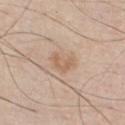Assessment: The lesion was photographed on a routine skin check and not biopsied; there is no pathology result. Image and clinical context: The subject is a male aged 43 to 47. From the chest. Longest diameter approximately 3 mm. This is a white-light tile. A region of skin cropped from a whole-body photographic capture, roughly 15 mm wide.